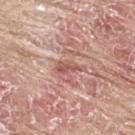The lesion was tiled from a total-body skin photograph and was not biopsied.